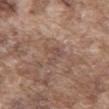Findings:
- workup: catalogued during a skin exam; not biopsied
- lesion diameter: about 2.5 mm
- patient: male, in their mid- to late 70s
- illumination: white-light
- TBP lesion metrics: a nevus-likeness score of about 0/100
- image source: ~15 mm tile from a whole-body skin photo
- anatomic site: the mid back